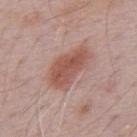No biopsy was performed on this lesion — it was imaged during a full skin examination and was not determined to be concerning.
Located on the mid back.
Captured under white-light illumination.
Cropped from a total-body skin-imaging series; the visible field is about 15 mm.
The patient is a male in their 70s.
The recorded lesion diameter is about 6 mm.